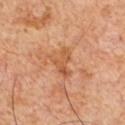location — the chest | image source — ~15 mm crop, total-body skin-cancer survey | illumination — cross-polarized | subject — male, roughly 60 years of age.